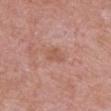Assessment:
The lesion was photographed on a routine skin check and not biopsied; there is no pathology result.